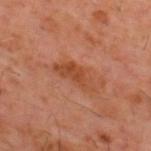A region of skin cropped from a whole-body photographic capture, roughly 15 mm wide.
A male patient approximately 60 years of age.
Located on the upper back.